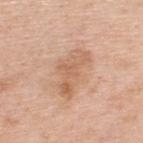Q: Was a biopsy performed?
A: total-body-photography surveillance lesion; no biopsy
Q: What is the anatomic site?
A: the upper back
Q: Who is the patient?
A: male, in their 50s
Q: How was this image acquired?
A: ~15 mm crop, total-body skin-cancer survey